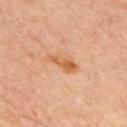Notes:
* subject: male, aged around 60
* location: the back
* image: total-body-photography crop, ~15 mm field of view